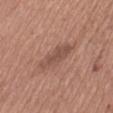notes: catalogued during a skin exam; not biopsied | patient: female, aged 53–57 | site: the back | acquisition: ~15 mm crop, total-body skin-cancer survey.